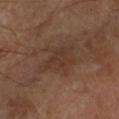Q: Was a biopsy performed?
A: no biopsy performed (imaged during a skin exam)
Q: What kind of image is this?
A: total-body-photography crop, ~15 mm field of view
Q: How was the tile lit?
A: cross-polarized
Q: Who is the patient?
A: male, about 65 years old
Q: What is the anatomic site?
A: the left lower leg